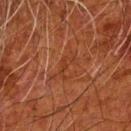biopsy_status: not biopsied; imaged during a skin examination
lighting: cross-polarized
lesion_size:
  long_diameter_mm_approx: 3.0
automated_metrics:
  area_mm2_approx: 2.5
  eccentricity: 0.9
  border_irregularity_0_10: 7.0
  color_variation_0_10: 0.0
  peripheral_color_asymmetry: 0.0
  nevus_likeness_0_100: 0
patient:
  sex: male
  age_approx: 80
image:
  source: total-body photography crop
  field_of_view_mm: 15
site: left forearm